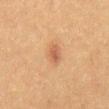Assessment: Part of a total-body skin-imaging series; this lesion was reviewed on a skin check and was not flagged for biopsy. Context: A 15 mm close-up tile from a total-body photography series done for melanoma screening. Measured at roughly 2.5 mm in maximum diameter. The lesion is on the abdomen. A male patient, aged approximately 50.